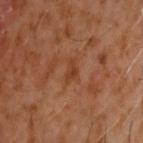Clinical summary: Cropped from a total-body skin-imaging series; the visible field is about 15 mm. Imaged with cross-polarized lighting. Automated image analysis of the tile measured a footprint of about 3 mm², an outline eccentricity of about 0.85 (0 = round, 1 = elongated), and a symmetry-axis asymmetry near 0.4. And it measured an average lesion color of about L≈37 a*≈23 b*≈33 (CIELAB), roughly 6 lightness units darker than nearby skin, and a normalized lesion–skin contrast near 6. The software also gave a nevus-likeness score of about 0/100 and a lesion-detection confidence of about 100/100. On the head or neck. The patient is a male aged 58–62. The recorded lesion diameter is about 2.5 mm.